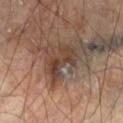Impression:
The lesion was tiled from a total-body skin photograph and was not biopsied.
Context:
About 5 mm across. Located on the leg. An algorithmic analysis of the crop reported a border-irregularity index near 5.5/10 and a peripheral color-asymmetry measure near 1.5. A male subject, aged around 65. A 15 mm crop from a total-body photograph taken for skin-cancer surveillance.